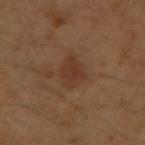No biopsy was performed on this lesion — it was imaged during a full skin examination and was not determined to be concerning. This image is a 15 mm lesion crop taken from a total-body photograph. The lesion is on the right upper arm. A male subject about 45 years old.